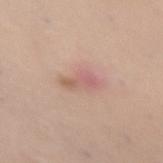biopsy_status: not biopsied; imaged during a skin examination
lighting: white-light
automated_metrics:
  nevus_likeness_0_100: 0
  lesion_detection_confidence_0_100: 100
patient:
  sex: female
  age_approx: 45
lesion_size:
  long_diameter_mm_approx: 4.0
site: front of the torso
image:
  source: total-body photography crop
  field_of_view_mm: 15